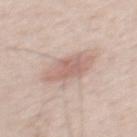<record>
  <biopsy_status>not biopsied; imaged during a skin examination</biopsy_status>
  <patient>
    <sex>male</sex>
    <age_approx>40</age_approx>
  </patient>
  <image>
    <source>total-body photography crop</source>
    <field_of_view_mm>15</field_of_view_mm>
  </image>
  <site>mid back</site>
</record>